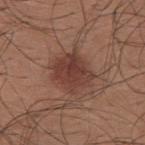The lesion was photographed on a routine skin check and not biopsied; there is no pathology result. A close-up tile cropped from a whole-body skin photograph, about 15 mm across. The lesion is on the upper back. A male subject about 25 years old.